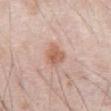| feature | finding |
|---|---|
| workup | no biopsy performed (imaged during a skin exam) |
| site | the abdomen |
| subject | male, aged approximately 70 |
| imaging modality | 15 mm crop, total-body photography |